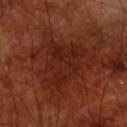subject = male, aged approximately 70 | acquisition = ~15 mm tile from a whole-body skin photo | image-analysis metrics = a footprint of about 20 mm² and two-axis asymmetry of about 0.45; a classifier nevus-likeness of about 0/100 and lesion-presence confidence of about 95/100 | location = the front of the torso.